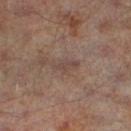No biopsy was performed on this lesion — it was imaged during a full skin examination and was not determined to be concerning. A male subject approximately 65 years of age. This is a cross-polarized tile. Longest diameter approximately 3 mm. Cropped from a total-body skin-imaging series; the visible field is about 15 mm. Located on the left thigh.